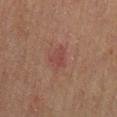No biopsy was performed on this lesion — it was imaged during a full skin examination and was not determined to be concerning.
The patient is a male aged around 70.
About 3.5 mm across.
This image is a 15 mm lesion crop taken from a total-body photograph.
On the mid back.
Imaged with cross-polarized lighting.